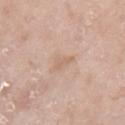No biopsy was performed on this lesion — it was imaged during a full skin examination and was not determined to be concerning.
A roughly 15 mm field-of-view crop from a total-body skin photograph.
From the right upper arm.
A female patient, approximately 70 years of age.
Captured under white-light illumination.
About 2.5 mm across.
The total-body-photography lesion software estimated a mean CIELAB color near L≈64 a*≈17 b*≈30 and a normalized border contrast of about 5. It also reported a border-irregularity index near 3.5/10, a color-variation rating of about 0/10, and radial color variation of about 0. It also reported a classifier nevus-likeness of about 0/100 and lesion-presence confidence of about 100/100.